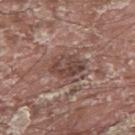| feature | finding |
|---|---|
| biopsy status | total-body-photography surveillance lesion; no biopsy |
| patient | male, aged around 40 |
| anatomic site | the upper back |
| image | 15 mm crop, total-body photography |
| lighting | white-light |
| lesion diameter | ≈4 mm |